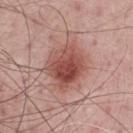Clinical impression: Imaged during a routine full-body skin examination; the lesion was not biopsied and no histopathology is available. Context: A male subject, in their mid- to late 60s. Imaged with white-light lighting. Located on the chest. A 15 mm close-up extracted from a 3D total-body photography capture.